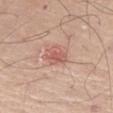Assessment:
The lesion was photographed on a routine skin check and not biopsied; there is no pathology result.
Clinical summary:
Cropped from a whole-body photographic skin survey; the tile spans about 15 mm. From the right thigh. About 3 mm across. The tile uses white-light illumination. A male patient approximately 80 years of age. Automated tile analysis of the lesion measured a footprint of about 5 mm². The software also gave a classifier nevus-likeness of about 35/100 and lesion-presence confidence of about 100/100.